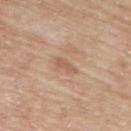Captured during whole-body skin photography for melanoma surveillance; the lesion was not biopsied.